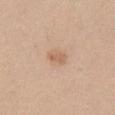The lesion was photographed on a routine skin check and not biopsied; there is no pathology result.
Located on the left thigh.
The patient is a female roughly 40 years of age.
Approximately 2.5 mm at its widest.
Captured under white-light illumination.
A region of skin cropped from a whole-body photographic capture, roughly 15 mm wide.
The lesion-visualizer software estimated a footprint of about 3 mm² and two-axis asymmetry of about 0.3. The analysis additionally found a nevus-likeness score of about 15/100 and a detector confidence of about 100 out of 100 that the crop contains a lesion.